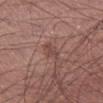{"biopsy_status": "not biopsied; imaged during a skin examination", "image": {"source": "total-body photography crop", "field_of_view_mm": 15}, "patient": {"sex": "male", "age_approx": 45}, "site": "left lower leg"}